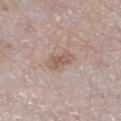biopsy status: catalogued during a skin exam; not biopsied | subject: female, in their 40s | lesion size: about 3 mm | image source: ~15 mm crop, total-body skin-cancer survey | tile lighting: white-light illumination | location: the leg.